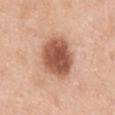Impression:
Part of a total-body skin-imaging series; this lesion was reviewed on a skin check and was not flagged for biopsy.
Context:
Cropped from a whole-body photographic skin survey; the tile spans about 15 mm. On the chest. The subject is a female aged 38 to 42. The recorded lesion diameter is about 5.5 mm.